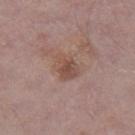Impression:
The lesion was tiled from a total-body skin photograph and was not biopsied.
Clinical summary:
The tile uses white-light illumination. Automated tile analysis of the lesion measured a lesion–skin lightness drop of about 8 and a normalized border contrast of about 6.5. The software also gave internal color variation of about 3.5 on a 0–10 scale and peripheral color asymmetry of about 1. It also reported a classifier nevus-likeness of about 0/100. Cropped from a whole-body photographic skin survey; the tile spans about 15 mm. On the right thigh. A male subject, in their mid-70s. About 3.5 mm across.